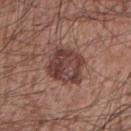This lesion was catalogued during total-body skin photography and was not selected for biopsy. Longest diameter approximately 5 mm. This image is a 15 mm lesion crop taken from a total-body photograph. Located on the right upper arm. Imaged with white-light lighting. An algorithmic analysis of the crop reported an area of roughly 15 mm², an eccentricity of roughly 0.5, and a shape-asymmetry score of about 0.15 (0 = symmetric). The software also gave a nevus-likeness score of about 20/100 and lesion-presence confidence of about 100/100. A male patient in their mid- to late 60s.